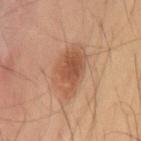{
  "biopsy_status": "not biopsied; imaged during a skin examination",
  "image": {
    "source": "total-body photography crop",
    "field_of_view_mm": 15
  },
  "patient": {
    "sex": "male",
    "age_approx": 55
  },
  "site": "mid back"
}